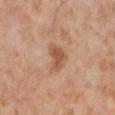Recorded during total-body skin imaging; not selected for excision or biopsy. A lesion tile, about 15 mm wide, cut from a 3D total-body photograph. On the left lower leg. Captured under cross-polarized illumination. A female subject, aged 53–57.